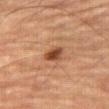Impression: The lesion was photographed on a routine skin check and not biopsied; there is no pathology result. Acquisition and patient details: A male subject, roughly 85 years of age or older. The lesion is on the abdomen. A lesion tile, about 15 mm wide, cut from a 3D total-body photograph. The lesion's longest dimension is about 2.5 mm. Captured under cross-polarized illumination. Automated tile analysis of the lesion measured an average lesion color of about L≈40 a*≈23 b*≈31 (CIELAB), a lesion–skin lightness drop of about 12, and a lesion-to-skin contrast of about 10 (normalized; higher = more distinct). The analysis additionally found a lesion-detection confidence of about 100/100.